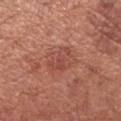Clinical impression: This lesion was catalogued during total-body skin photography and was not selected for biopsy. Acquisition and patient details: Captured under white-light illumination. A close-up tile cropped from a whole-body skin photograph, about 15 mm across. Measured at roughly 3.5 mm in maximum diameter. The lesion is located on the left forearm. The subject is a female aged around 50.